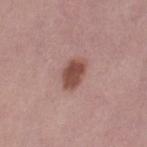Findings:
- notes · total-body-photography surveillance lesion; no biopsy
- tile lighting · white-light illumination
- site · the left thigh
- image-analysis metrics · an area of roughly 7 mm² and an eccentricity of roughly 0.8; a lesion–skin lightness drop of about 13 and a normalized border contrast of about 9.5; a border-irregularity rating of about 2/10, a within-lesion color-variation index near 3/10, and peripheral color asymmetry of about 1
- acquisition · ~15 mm crop, total-body skin-cancer survey
- diameter · ≈3.5 mm
- subject · female, aged 33–37